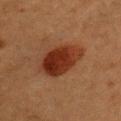biopsy_status: not biopsied; imaged during a skin examination